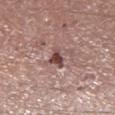This lesion was catalogued during total-body skin photography and was not selected for biopsy.
Imaged with white-light lighting.
The recorded lesion diameter is about 3 mm.
The subject is a male aged 53–57.
The lesion is located on the right lower leg.
Cropped from a whole-body photographic skin survey; the tile spans about 15 mm.